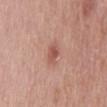The lesion was tiled from a total-body skin photograph and was not biopsied. A roughly 15 mm field-of-view crop from a total-body skin photograph. The recorded lesion diameter is about 2.5 mm. The lesion is on the mid back. A male subject, aged 63–67. This is a white-light tile.